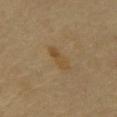Imaged during a routine full-body skin examination; the lesion was not biopsied and no histopathology is available.
The recorded lesion diameter is about 3.5 mm.
From the mid back.
A male subject, approximately 65 years of age.
The total-body-photography lesion software estimated a footprint of about 4.5 mm² and an eccentricity of roughly 0.95. And it measured a lesion–skin lightness drop of about 6 and a lesion-to-skin contrast of about 6.5 (normalized; higher = more distinct). The software also gave a lesion-detection confidence of about 100/100.
A lesion tile, about 15 mm wide, cut from a 3D total-body photograph.
The tile uses cross-polarized illumination.